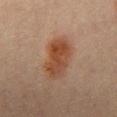<case>
<lighting>cross-polarized</lighting>
<patient>
  <sex>male</sex>
  <age_approx>70</age_approx>
</patient>
<lesion_size>
  <long_diameter_mm_approx>6.0</long_diameter_mm_approx>
</lesion_size>
<site>mid back</site>
<image>
  <source>total-body photography crop</source>
  <field_of_view_mm>15</field_of_view_mm>
</image>
</case>